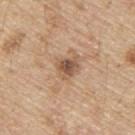Captured during whole-body skin photography for melanoma surveillance; the lesion was not biopsied.
The subject is a male roughly 70 years of age.
Imaged with white-light lighting.
The lesion is on the back.
Automated image analysis of the tile measured an area of roughly 5 mm², a shape eccentricity near 0.5, and a symmetry-axis asymmetry near 0.4. The analysis additionally found border irregularity of about 3.5 on a 0–10 scale, internal color variation of about 5.5 on a 0–10 scale, and radial color variation of about 1.5. The analysis additionally found a nevus-likeness score of about 55/100.
This image is a 15 mm lesion crop taken from a total-body photograph.